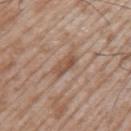workup = imaged on a skin check; not biopsied | lesion diameter = about 3.5 mm | acquisition = total-body-photography crop, ~15 mm field of view | patient = male, aged 48 to 52 | site = the arm.